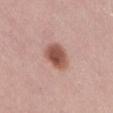The lesion was tiled from a total-body skin photograph and was not biopsied.